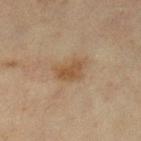Notes:
* notes: no biopsy performed (imaged during a skin exam)
* size: ~4 mm (longest diameter)
* patient: female, aged 53–57
* body site: the left lower leg
* image: ~15 mm tile from a whole-body skin photo
* lighting: cross-polarized